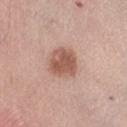Measured at roughly 3.5 mm in maximum diameter. A roughly 15 mm field-of-view crop from a total-body skin photograph. Imaged with white-light lighting. The lesion is on the right lower leg. A female subject in their mid- to late 60s. An algorithmic analysis of the crop reported an area of roughly 11 mm², a shape eccentricity near 0.4, and a symmetry-axis asymmetry near 0.2. And it measured a lesion color around L≈56 a*≈22 b*≈28 in CIELAB and a normalized lesion–skin contrast near 8. The analysis additionally found a border-irregularity index near 1.5/10, a color-variation rating of about 3.5/10, and a peripheral color-asymmetry measure near 1. The analysis additionally found a nevus-likeness score of about 90/100 and a detector confidence of about 100 out of 100 that the crop contains a lesion.